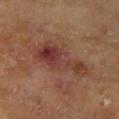A roughly 15 mm field-of-view crop from a total-body skin photograph. A female subject aged 78 to 82. Imaged with cross-polarized lighting. On the right forearm.